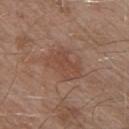biopsy status: no biopsy performed (imaged during a skin exam) | image source: ~15 mm crop, total-body skin-cancer survey | anatomic site: the right upper arm | subject: male, approximately 55 years of age | lighting: white-light illumination.